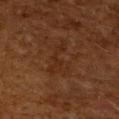Findings:
– workup — catalogued during a skin exam; not biopsied
– lesion size — ≈5 mm
– image — 15 mm crop, total-body photography
– image-analysis metrics — a lesion area of about 8 mm², a shape eccentricity near 0.9, and two-axis asymmetry of about 0.65; lesion-presence confidence of about 85/100
– illumination — cross-polarized illumination
– patient — male, aged 58 to 62
– location — the left upper arm